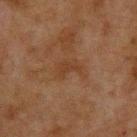Assessment:
No biopsy was performed on this lesion — it was imaged during a full skin examination and was not determined to be concerning.
Clinical summary:
A 15 mm crop from a total-body photograph taken for skin-cancer surveillance. This is a cross-polarized tile. The lesion-visualizer software estimated a shape eccentricity near 0.8 and a symmetry-axis asymmetry near 0.45. And it measured an average lesion color of about L≈29 a*≈16 b*≈25 (CIELAB) and about 4 CIELAB-L* units darker than the surrounding skin. It also reported a border-irregularity rating of about 5.5/10, internal color variation of about 1 on a 0–10 scale, and radial color variation of about 0.5. The analysis additionally found a nevus-likeness score of about 0/100 and a lesion-detection confidence of about 100/100. A male subject approximately 60 years of age. The lesion's longest dimension is about 3.5 mm. The lesion is on the upper back.